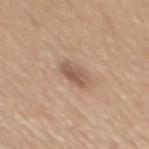Q: Is there a histopathology result?
A: total-body-photography surveillance lesion; no biopsy
Q: Where on the body is the lesion?
A: the mid back
Q: Patient demographics?
A: male, in their 50s
Q: What is the imaging modality?
A: 15 mm crop, total-body photography
Q: Illumination type?
A: white-light
Q: What is the lesion's diameter?
A: about 3 mm